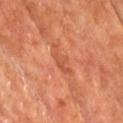image-analysis metrics: an area of roughly 5 mm² and an outline eccentricity of about 0.9 (0 = round, 1 = elongated); border irregularity of about 5.5 on a 0–10 scale, internal color variation of about 2 on a 0–10 scale, and peripheral color asymmetry of about 0.5; an automated nevus-likeness rating near 0 out of 100 and a lesion-detection confidence of about 100/100
location: the back
patient: male, aged around 60
imaging modality: ~15 mm tile from a whole-body skin photo